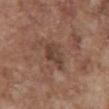Recorded during total-body skin imaging; not selected for excision or biopsy.
The lesion's longest dimension is about 3.5 mm.
This image is a 15 mm lesion crop taken from a total-body photograph.
The lesion is located on the chest.
A male patient aged 73–77.